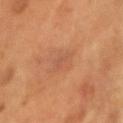No biopsy was performed on this lesion — it was imaged during a full skin examination and was not determined to be concerning.
Measured at roughly 2 mm in maximum diameter.
Imaged with cross-polarized lighting.
The lesion is located on the head or neck.
Cropped from a whole-body photographic skin survey; the tile spans about 15 mm.
A male patient in their mid- to late 50s.
The total-body-photography lesion software estimated an average lesion color of about L≈49 a*≈23 b*≈31 (CIELAB), about 5 CIELAB-L* units darker than the surrounding skin, and a normalized border contrast of about 4. It also reported a color-variation rating of about 0.5/10 and peripheral color asymmetry of about 0. It also reported a nevus-likeness score of about 5/100 and a lesion-detection confidence of about 100/100.